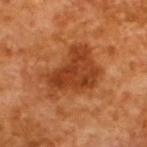Q: Was a biopsy performed?
A: no biopsy performed (imaged during a skin exam)
Q: Illumination type?
A: cross-polarized illumination
Q: How was this image acquired?
A: 15 mm crop, total-body photography
Q: Who is the patient?
A: male, roughly 65 years of age